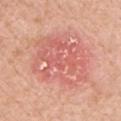follow-up: catalogued during a skin exam; not biopsied | image: ~15 mm crop, total-body skin-cancer survey | illumination: white-light illumination | TBP lesion metrics: a lesion area of about 30 mm², an outline eccentricity of about 0.65 (0 = round, 1 = elongated), and a shape-asymmetry score of about 0.25 (0 = symmetric); an average lesion color of about L≈62 a*≈30 b*≈29 (CIELAB); a peripheral color-asymmetry measure near 2; a detector confidence of about 100 out of 100 that the crop contains a lesion | body site: the front of the torso | patient: male, aged around 60.